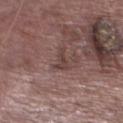Cropped from a total-body skin-imaging series; the visible field is about 15 mm.
A male subject aged 73 to 77.
The lesion's longest dimension is about 3.5 mm.
From the left lower leg.